A male patient, roughly 75 years of age. A roughly 15 mm field-of-view crop from a total-body skin photograph. From the left lower leg:
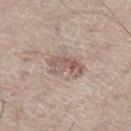{"lighting": "white-light", "diagnosis": {"histopathology": "nodular basal cell carcinoma", "malignancy": "malignant", "taxonomic_path": ["Malignant", "Malignant adnexal epithelial proliferations - Follicular", "Basal cell carcinoma", "Basal cell carcinoma, Nodular"]}}